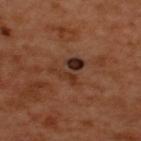The patient is a male aged 48 to 52. A 15 mm close-up extracted from a 3D total-body photography capture. The total-body-photography lesion software estimated a footprint of about 6 mm² and an outline eccentricity of about 0.65 (0 = round, 1 = elongated). The analysis additionally found a border-irregularity index near 7.5/10, internal color variation of about 6 on a 0–10 scale, and a peripheral color-asymmetry measure near 2. On the upper back. The lesion's longest dimension is about 4 mm.